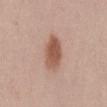This lesion was catalogued during total-body skin photography and was not selected for biopsy. A region of skin cropped from a whole-body photographic capture, roughly 15 mm wide. A female patient, roughly 40 years of age. Imaged with white-light lighting. Measured at roughly 4 mm in maximum diameter. The lesion is located on the back. The lesion-visualizer software estimated a lesion–skin lightness drop of about 12 and a normalized lesion–skin contrast near 8.5.